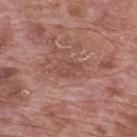From the back.
A male subject, roughly 70 years of age.
A close-up tile cropped from a whole-body skin photograph, about 15 mm across.
About 3.5 mm across.